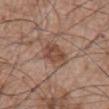Acquisition and patient details: The patient is a male aged around 65. This is a white-light tile. Cropped from a total-body skin-imaging series; the visible field is about 15 mm. About 4 mm across. From the chest.